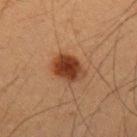Notes:
* workup: total-body-photography surveillance lesion; no biopsy
* image-analysis metrics: a lesion–skin lightness drop of about 13 and a normalized border contrast of about 11; a classifier nevus-likeness of about 100/100
* imaging modality: ~15 mm tile from a whole-body skin photo
* location: the left upper arm
* patient: male, aged approximately 35
* size: about 4 mm
* illumination: cross-polarized illumination